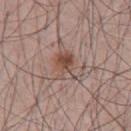Q: Was this lesion biopsied?
A: catalogued during a skin exam; not biopsied
Q: What kind of image is this?
A: 15 mm crop, total-body photography
Q: How was the tile lit?
A: white-light illumination
Q: What is the anatomic site?
A: the chest
Q: Patient demographics?
A: male, in their mid-60s